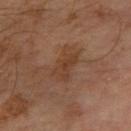| feature | finding |
|---|---|
| image source | total-body-photography crop, ~15 mm field of view |
| patient | male, roughly 60 years of age |
| illumination | cross-polarized illumination |
| location | the left forearm |
| diameter | ~4.5 mm (longest diameter) |